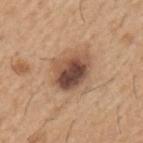No biopsy was performed on this lesion — it was imaged during a full skin examination and was not determined to be concerning.
The lesion is located on the left upper arm.
The patient is a male roughly 65 years of age.
A 15 mm close-up tile from a total-body photography series done for melanoma screening.